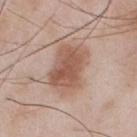Q: Was a biopsy performed?
A: imaged on a skin check; not biopsied
Q: How was this image acquired?
A: total-body-photography crop, ~15 mm field of view
Q: Automated lesion metrics?
A: about 11 CIELAB-L* units darker than the surrounding skin and a normalized border contrast of about 8; an automated nevus-likeness rating near 85 out of 100 and a detector confidence of about 100 out of 100 that the crop contains a lesion
Q: Illumination type?
A: white-light
Q: What are the patient's age and sex?
A: male, aged 53–57
Q: Where on the body is the lesion?
A: the front of the torso